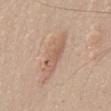This lesion was catalogued during total-body skin photography and was not selected for biopsy.
The subject is a male approximately 65 years of age.
On the chest.
A close-up tile cropped from a whole-body skin photograph, about 15 mm across.